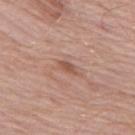Assessment:
Imaged during a routine full-body skin examination; the lesion was not biopsied and no histopathology is available.
Context:
A female subject about 70 years old. The tile uses white-light illumination. A 15 mm close-up tile from a total-body photography series done for melanoma screening. The lesion's longest dimension is about 3 mm. The lesion is on the left thigh.